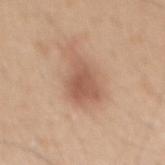Findings:
– workup — imaged on a skin check; not biopsied
– subject — male, about 45 years old
– imaging modality — ~15 mm tile from a whole-body skin photo
– body site — the mid back
– illumination — white-light
– lesion diameter — about 5 mm